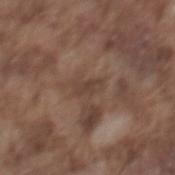| field | value |
|---|---|
| follow-up | imaged on a skin check; not biopsied |
| site | the mid back |
| illumination | white-light |
| image source | 15 mm crop, total-body photography |
| subject | male, aged 73 to 77 |
| image-analysis metrics | a footprint of about 3 mm² and an outline eccentricity of about 0.9 (0 = round, 1 = elongated); a mean CIELAB color near L≈40 a*≈16 b*≈23, roughly 7 lightness units darker than nearby skin, and a normalized lesion–skin contrast near 6.5; a border-irregularity rating of about 5/10 and a peripheral color-asymmetry measure near 0; an automated nevus-likeness rating near 0 out of 100 and a detector confidence of about 60 out of 100 that the crop contains a lesion |